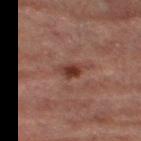Q: Was this lesion biopsied?
A: total-body-photography surveillance lesion; no biopsy
Q: Illumination type?
A: cross-polarized illumination
Q: Lesion location?
A: the right thigh
Q: What kind of image is this?
A: ~15 mm tile from a whole-body skin photo
Q: Who is the patient?
A: female, in their 70s
Q: What did automated image analysis measure?
A: an area of roughly 3.5 mm², an outline eccentricity of about 0.75 (0 = round, 1 = elongated), and two-axis asymmetry of about 0.25; internal color variation of about 2.5 on a 0–10 scale